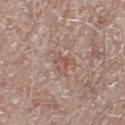– notes — no biopsy performed (imaged during a skin exam)
– illumination — white-light illumination
– automated metrics — an automated nevus-likeness rating near 0 out of 100
– imaging modality — 15 mm crop, total-body photography
– diameter — ≈2.5 mm
– subject — male, in their 80s
– location — the leg